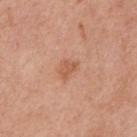notes = catalogued during a skin exam; not biopsied
image source = 15 mm crop, total-body photography
body site = the chest
patient = male, roughly 70 years of age